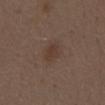biopsy status: no biopsy performed (imaged during a skin exam)
lesion diameter: about 3 mm
acquisition: ~15 mm tile from a whole-body skin photo
lighting: white-light illumination
image-analysis metrics: a footprint of about 4.5 mm² and two-axis asymmetry of about 0.25; a border-irregularity rating of about 2.5/10 and a within-lesion color-variation index near 2/10; a nevus-likeness score of about 80/100 and a detector confidence of about 100 out of 100 that the crop contains a lesion
subject: male, aged 68 to 72
location: the mid back